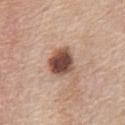Clinical impression: The lesion was photographed on a routine skin check and not biopsied; there is no pathology result. Acquisition and patient details: About 3.5 mm across. A female subject, about 60 years old. Imaged with white-light lighting. The lesion is on the chest. This image is a 15 mm lesion crop taken from a total-body photograph.